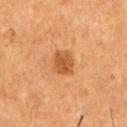- follow-up — no biopsy performed (imaged during a skin exam)
- automated lesion analysis — a mean CIELAB color near L≈51 a*≈25 b*≈39, about 11 CIELAB-L* units darker than the surrounding skin, and a normalized border contrast of about 7.5; a border-irregularity rating of about 1.5/10, a color-variation rating of about 3/10, and radial color variation of about 1
- patient — male, aged 53 to 57
- location — the chest
- imaging modality — ~15 mm crop, total-body skin-cancer survey